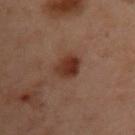{"biopsy_status": "not biopsied; imaged during a skin examination", "lesion_size": {"long_diameter_mm_approx": 3.5}, "lighting": "cross-polarized", "patient": {"sex": "female", "age_approx": 55}, "site": "left arm", "image": {"source": "total-body photography crop", "field_of_view_mm": 15}}